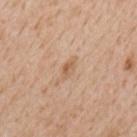The lesion was tiled from a total-body skin photograph and was not biopsied.